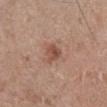Captured during whole-body skin photography for melanoma surveillance; the lesion was not biopsied.
The lesion-visualizer software estimated a lesion area of about 4.5 mm², an eccentricity of roughly 0.55, and a symmetry-axis asymmetry near 0.3. It also reported roughly 10 lightness units darker than nearby skin.
A male subject in their mid- to late 50s.
A 15 mm close-up tile from a total-body photography series done for melanoma screening.
From the leg.
The tile uses white-light illumination.
Measured at roughly 2.5 mm in maximum diameter.